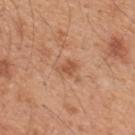Q: Was this lesion biopsied?
A: no biopsy performed (imaged during a skin exam)
Q: Automated lesion metrics?
A: a footprint of about 3 mm², an outline eccentricity of about 0.8 (0 = round, 1 = elongated), and two-axis asymmetry of about 0.35; a lesion color around L≈54 a*≈25 b*≈34 in CIELAB and a normalized border contrast of about 6.5; a border-irregularity rating of about 3.5/10 and a within-lesion color-variation index near 0.5/10; a detector confidence of about 100 out of 100 that the crop contains a lesion
Q: Illumination type?
A: white-light illumination
Q: Lesion location?
A: the upper back
Q: Patient demographics?
A: male, in their mid- to late 40s
Q: What is the lesion's diameter?
A: ~2.5 mm (longest diameter)
Q: How was this image acquired?
A: 15 mm crop, total-body photography